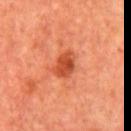A male subject in their mid- to late 60s. Approximately 3.5 mm at its widest. Cropped from a total-body skin-imaging series; the visible field is about 15 mm. Located on the chest.